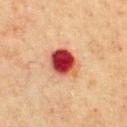follow-up = imaged on a skin check; not biopsied
location = the chest
automated metrics = an area of roughly 11 mm², a shape eccentricity near 0.55, and two-axis asymmetry of about 0.2; an automated nevus-likeness rating near 0 out of 100 and a lesion-detection confidence of about 100/100
patient = male, about 60 years old
image source = ~15 mm tile from a whole-body skin photo